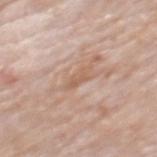Captured during whole-body skin photography for melanoma surveillance; the lesion was not biopsied. On the mid back. The patient is a male aged 78 to 82. Captured under white-light illumination. A lesion tile, about 15 mm wide, cut from a 3D total-body photograph.